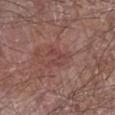{"biopsy_status": "not biopsied; imaged during a skin examination", "patient": {"sex": "male", "age_approx": 80}, "site": "left forearm", "image": {"source": "total-body photography crop", "field_of_view_mm": 15}}